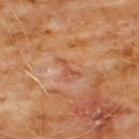<case>
<biopsy_status>not biopsied; imaged during a skin examination</biopsy_status>
<image>
  <source>total-body photography crop</source>
  <field_of_view_mm>15</field_of_view_mm>
</image>
<lesion_size>
  <long_diameter_mm_approx>2.5</long_diameter_mm_approx>
</lesion_size>
<patient>
  <sex>male</sex>
  <age_approx>60</age_approx>
</patient>
<site>chest</site>
</case>